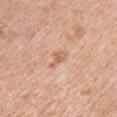The lesion was photographed on a routine skin check and not biopsied; there is no pathology result. Captured under white-light illumination. From the chest. A lesion tile, about 15 mm wide, cut from a 3D total-body photograph. A female patient aged approximately 40.